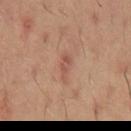workup: no biopsy performed (imaged during a skin exam); lighting: cross-polarized illumination; subject: male, approximately 60 years of age; image: ~15 mm crop, total-body skin-cancer survey; site: the back.